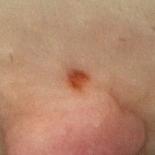Assessment:
Recorded during total-body skin imaging; not selected for excision or biopsy.
Context:
The lesion-visualizer software estimated an eccentricity of roughly 0.3 and two-axis asymmetry of about 0.2. The software also gave a border-irregularity index near 1.5/10 and peripheral color asymmetry of about 2. The analysis additionally found a nevus-likeness score of about 100/100 and lesion-presence confidence of about 100/100. Approximately 2.5 mm at its widest. On the chest. The subject is a female approximately 20 years of age. A 15 mm close-up tile from a total-body photography series done for melanoma screening.